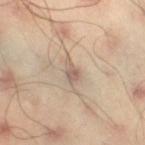Impression: Captured during whole-body skin photography for melanoma surveillance; the lesion was not biopsied. Clinical summary: A close-up tile cropped from a whole-body skin photograph, about 15 mm across. The lesion is on the right thigh. Automated tile analysis of the lesion measured an eccentricity of roughly 0.8 and a shape-asymmetry score of about 0.4 (0 = symmetric). The software also gave about 10 CIELAB-L* units darker than the surrounding skin and a normalized border contrast of about 7. It also reported a within-lesion color-variation index near 2/10. The analysis additionally found a nevus-likeness score of about 0/100 and a lesion-detection confidence of about 80/100. The lesion's longest dimension is about 2.5 mm. The subject is a male in their mid- to late 40s.